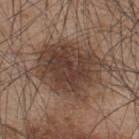Assessment:
Captured during whole-body skin photography for melanoma surveillance; the lesion was not biopsied.